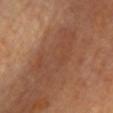Notes:
- notes: total-body-photography surveillance lesion; no biopsy
- lighting: cross-polarized
- subject: female, aged approximately 60
- imaging modality: ~15 mm crop, total-body skin-cancer survey
- body site: the chest